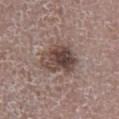• TBP lesion metrics · a lesion area of about 14 mm²; a nevus-likeness score of about 55/100 and a detector confidence of about 100 out of 100 that the crop contains a lesion
• lesion size · about 5 mm
• imaging modality · ~15 mm tile from a whole-body skin photo
• illumination · white-light
• site · the left lower leg
• subject · male, roughly 60 years of age